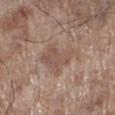Notes:
- follow-up: imaged on a skin check; not biopsied
- image source: ~15 mm tile from a whole-body skin photo
- diameter: about 5 mm
- illumination: white-light
- body site: the left lower leg
- automated metrics: a footprint of about 14 mm² and two-axis asymmetry of about 0.45
- subject: male, aged approximately 70